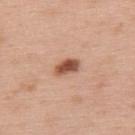Impression: This lesion was catalogued during total-body skin photography and was not selected for biopsy. Clinical summary: On the upper back. Cropped from a whole-body photographic skin survey; the tile spans about 15 mm. A male subject about 60 years old. About 3 mm across. Captured under white-light illumination.